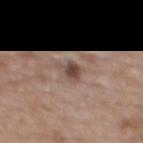This lesion was catalogued during total-body skin photography and was not selected for biopsy. A lesion tile, about 15 mm wide, cut from a 3D total-body photograph. The tile uses white-light illumination. Located on the upper back. About 3 mm across. A female subject approximately 60 years of age.